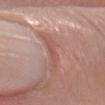Background:
An algorithmic analysis of the crop reported an average lesion color of about L≈54 a*≈23 b*≈24 (CIELAB), roughly 7 lightness units darker than nearby skin, and a normalized border contrast of about 5. Measured at roughly 3.5 mm in maximum diameter. The lesion is on the left forearm. Cropped from a whole-body photographic skin survey; the tile spans about 15 mm. A male subject aged 53 to 57.